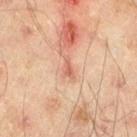| field | value |
|---|---|
| workup | catalogued during a skin exam; not biopsied |